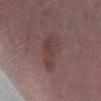The lesion was photographed on a routine skin check and not biopsied; there is no pathology result. A 15 mm close-up tile from a total-body photography series done for melanoma screening. The tile uses white-light illumination. A male patient, roughly 75 years of age. Measured at roughly 4.5 mm in maximum diameter. Automated tile analysis of the lesion measured a lesion–skin lightness drop of about 6 and a normalized lesion–skin contrast near 5.5. The analysis additionally found internal color variation of about 2 on a 0–10 scale and a peripheral color-asymmetry measure near 0.5. Located on the left lower leg.